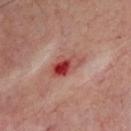The lesion was tiled from a total-body skin photograph and was not biopsied. A male subject aged 48–52. A roughly 15 mm field-of-view crop from a total-body skin photograph. From the upper back. The lesion's longest dimension is about 4.5 mm. This is a cross-polarized tile.